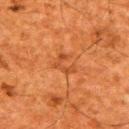Part of a total-body skin-imaging series; this lesion was reviewed on a skin check and was not flagged for biopsy.
About 3 mm across.
The lesion is on the upper back.
A close-up tile cropped from a whole-body skin photograph, about 15 mm across.
A male patient roughly 60 years of age.
This is a cross-polarized tile.